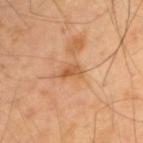Impression:
The lesion was tiled from a total-body skin photograph and was not biopsied.
Clinical summary:
Longest diameter approximately 2.5 mm. The lesion is on the upper back. A 15 mm close-up extracted from a 3D total-body photography capture. The total-body-photography lesion software estimated an average lesion color of about L≈57 a*≈24 b*≈40 (CIELAB), about 10 CIELAB-L* units darker than the surrounding skin, and a normalized lesion–skin contrast near 7. It also reported a border-irregularity rating of about 3.5/10 and a peripheral color-asymmetry measure near 1. The analysis additionally found a nevus-likeness score of about 0/100 and lesion-presence confidence of about 100/100. This is a cross-polarized tile. A male subject in their 40s.